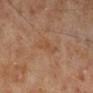Clinical summary: The tile uses cross-polarized illumination. Measured at roughly 3.5 mm in maximum diameter. A male patient approximately 70 years of age. A region of skin cropped from a whole-body photographic capture, roughly 15 mm wide. On the right lower leg.